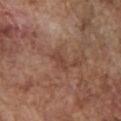Q: Is there a histopathology result?
A: no biopsy performed (imaged during a skin exam)
Q: What are the patient's age and sex?
A: male, aged approximately 75
Q: Lesion size?
A: ≈2.5 mm
Q: What is the imaging modality?
A: total-body-photography crop, ~15 mm field of view
Q: Where on the body is the lesion?
A: the front of the torso